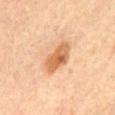Imaged during a routine full-body skin examination; the lesion was not biopsied and no histopathology is available.
Automated tile analysis of the lesion measured an outline eccentricity of about 0.8 (0 = round, 1 = elongated) and a symmetry-axis asymmetry near 0.25. And it measured a mean CIELAB color near L≈61 a*≈23 b*≈39, roughly 13 lightness units darker than nearby skin, and a normalized lesion–skin contrast near 8.5. The analysis additionally found a border-irregularity index near 2.5/10 and peripheral color asymmetry of about 1.5.
The lesion is located on the abdomen.
Captured under cross-polarized illumination.
A close-up tile cropped from a whole-body skin photograph, about 15 mm across.
Approximately 4 mm at its widest.
A male patient aged 63–67.